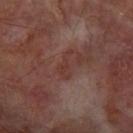The lesion was tiled from a total-body skin photograph and was not biopsied.
The tile uses cross-polarized illumination.
A region of skin cropped from a whole-body photographic capture, roughly 15 mm wide.
The subject is a male aged around 65.
The lesion is located on the arm.
Longest diameter approximately 3.5 mm.